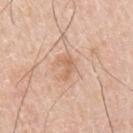<record>
<biopsy_status>not biopsied; imaged during a skin examination</biopsy_status>
<patient>
  <sex>male</sex>
  <age_approx>65</age_approx>
</patient>
<site>arm</site>
<image>
  <source>total-body photography crop</source>
  <field_of_view_mm>15</field_of_view_mm>
</image>
</record>